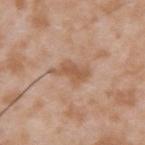Impression: Part of a total-body skin-imaging series; this lesion was reviewed on a skin check and was not flagged for biopsy. Clinical summary: Approximately 4 mm at its widest. A 15 mm crop from a total-body photograph taken for skin-cancer surveillance. A male subject, in their mid-30s. An algorithmic analysis of the crop reported a border-irregularity index near 5/10, a within-lesion color-variation index near 1.5/10, and a peripheral color-asymmetry measure near 0.5. The software also gave a classifier nevus-likeness of about 0/100 and lesion-presence confidence of about 100/100. From the upper back. This is a white-light tile.